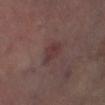The lesion was photographed on a routine skin check and not biopsied; there is no pathology result. This is a cross-polarized tile. Cropped from a total-body skin-imaging series; the visible field is about 15 mm. Longest diameter approximately 3 mm. The subject is a male aged 63 to 67. The lesion is located on the left lower leg. The lesion-visualizer software estimated a mean CIELAB color near L≈32 a*≈19 b*≈16, a lesion–skin lightness drop of about 6, and a lesion-to-skin contrast of about 6 (normalized; higher = more distinct). And it measured a classifier nevus-likeness of about 15/100 and a lesion-detection confidence of about 100/100.